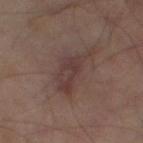This lesion was catalogued during total-body skin photography and was not selected for biopsy. Captured under cross-polarized illumination. The total-body-photography lesion software estimated an outline eccentricity of about 0.95 (0 = round, 1 = elongated) and two-axis asymmetry of about 0.4. It also reported a lesion color around L≈35 a*≈15 b*≈18 in CIELAB, a lesion–skin lightness drop of about 7, and a normalized border contrast of about 6.5. The analysis additionally found a border-irregularity rating of about 6/10 and a color-variation rating of about 3.5/10. The software also gave lesion-presence confidence of about 90/100. The recorded lesion diameter is about 6.5 mm. On the leg. A male subject aged 63–67. A roughly 15 mm field-of-view crop from a total-body skin photograph.